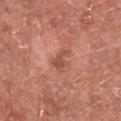The lesion was photographed on a routine skin check and not biopsied; there is no pathology result. The patient is a male aged around 75. A 15 mm crop from a total-body photograph taken for skin-cancer surveillance. The tile uses white-light illumination. Automated image analysis of the tile measured a border-irregularity index near 6/10 and a within-lesion color-variation index near 1/10. And it measured a classifier nevus-likeness of about 0/100 and a lesion-detection confidence of about 100/100. On the chest. Measured at roughly 3 mm in maximum diameter.